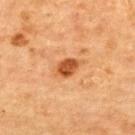follow-up: catalogued during a skin exam; not biopsied | imaging modality: ~15 mm tile from a whole-body skin photo | patient: female, roughly 70 years of age | anatomic site: the back | diameter: about 3 mm.